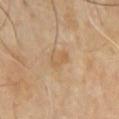Clinical impression: Imaged during a routine full-body skin examination; the lesion was not biopsied and no histopathology is available. Clinical summary: Cropped from a total-body skin-imaging series; the visible field is about 15 mm. From the chest. A male subject aged around 65.